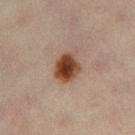The lesion was tiled from a total-body skin photograph and was not biopsied.
The tile uses cross-polarized illumination.
A close-up tile cropped from a whole-body skin photograph, about 15 mm across.
Automated tile analysis of the lesion measured a lesion color around L≈37 a*≈19 b*≈27 in CIELAB, about 14 CIELAB-L* units darker than the surrounding skin, and a normalized border contrast of about 13. It also reported a border-irregularity index near 2/10, internal color variation of about 4.5 on a 0–10 scale, and peripheral color asymmetry of about 1. The analysis additionally found a classifier nevus-likeness of about 100/100 and lesion-presence confidence of about 100/100.
The lesion is located on the left thigh.
Longest diameter approximately 3.5 mm.
The subject is a female aged 43 to 47.